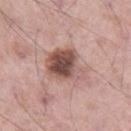Clinical impression:
Captured during whole-body skin photography for melanoma surveillance; the lesion was not biopsied.
Acquisition and patient details:
Imaged with white-light lighting. Located on the right thigh. A 15 mm crop from a total-body photograph taken for skin-cancer surveillance. A male subject aged 53–57. The total-body-photography lesion software estimated a border-irregularity rating of about 3/10 and a within-lesion color-variation index near 8/10. The software also gave a nevus-likeness score of about 60/100 and a detector confidence of about 100 out of 100 that the crop contains a lesion.